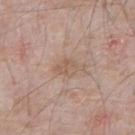* workup: no biopsy performed (imaged during a skin exam)
* location: the front of the torso
* subject: male, aged around 65
* image source: ~15 mm crop, total-body skin-cancer survey